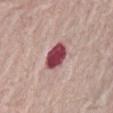Case summary:
- site — the abdomen
- image source — 15 mm crop, total-body photography
- lesion diameter — ~3.5 mm (longest diameter)
- subject — female, about 65 years old
- tile lighting — white-light illumination